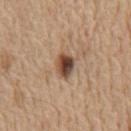The lesion was tiled from a total-body skin photograph and was not biopsied. Cropped from a whole-body photographic skin survey; the tile spans about 15 mm. A male subject, approximately 70 years of age. Imaged with white-light lighting. The lesion is located on the chest.